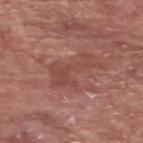biopsy_status: not biopsied; imaged during a skin examination
site: upper back
lighting: white-light
image:
  source: total-body photography crop
  field_of_view_mm: 15
lesion_size:
  long_diameter_mm_approx: 6.0
patient:
  sex: male
  age_approx: 75